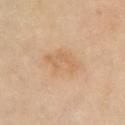workup: catalogued during a skin exam; not biopsied | image: ~15 mm tile from a whole-body skin photo | patient: female, in their mid-50s | diameter: ≈4.5 mm | lighting: cross-polarized | anatomic site: the chest | TBP lesion metrics: an area of roughly 8 mm², an eccentricity of roughly 0.8, and a shape-asymmetry score of about 0.4 (0 = symmetric); a lesion color around L≈65 a*≈19 b*≈37 in CIELAB, about 7 CIELAB-L* units darker than the surrounding skin, and a normalized lesion–skin contrast near 5; internal color variation of about 2 on a 0–10 scale and a peripheral color-asymmetry measure near 0.5; a nevus-likeness score of about 10/100.